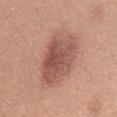biopsy status — total-body-photography surveillance lesion; no biopsy